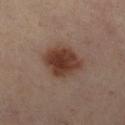No biopsy was performed on this lesion — it was imaged during a full skin examination and was not determined to be concerning.
A 15 mm crop from a total-body photograph taken for skin-cancer surveillance.
A female patient, aged around 40.
The total-body-photography lesion software estimated border irregularity of about 2 on a 0–10 scale and a within-lesion color-variation index near 4/10.
Longest diameter approximately 5 mm.
The tile uses cross-polarized illumination.
The lesion is on the right lower leg.